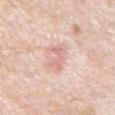Notes:
• follow-up: catalogued during a skin exam; not biopsied
• site: the chest
• automated lesion analysis: a lesion area of about 5.5 mm², a shape eccentricity near 0.9, and a symmetry-axis asymmetry near 0.35; a lesion–skin lightness drop of about 9
• subject: male, aged 63 to 67
• lighting: white-light illumination
• image source: total-body-photography crop, ~15 mm field of view
• lesion size: ≈4 mm